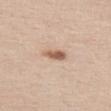Background:
A 15 mm close-up extracted from a 3D total-body photography capture. A male subject, about 60 years old. Located on the left thigh. The tile uses white-light illumination. The lesion's longest dimension is about 3 mm.